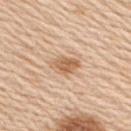Clinical summary:
A male subject approximately 60 years of age. The lesion is on the upper back. Longest diameter approximately 3.5 mm. Imaged with white-light lighting. Cropped from a total-body skin-imaging series; the visible field is about 15 mm.